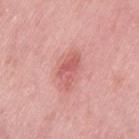The lesion was tiled from a total-body skin photograph and was not biopsied. Captured under white-light illumination. The lesion-visualizer software estimated a footprint of about 7 mm², an outline eccentricity of about 0.85 (0 = round, 1 = elongated), and a symmetry-axis asymmetry near 0.35. The software also gave roughly 10 lightness units darker than nearby skin and a normalized lesion–skin contrast near 6.5. It also reported a classifier nevus-likeness of about 40/100 and a lesion-detection confidence of about 100/100. A female subject aged approximately 40. A 15 mm close-up tile from a total-body photography series done for melanoma screening. From the left thigh. About 4.5 mm across.